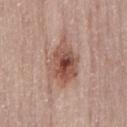workup = no biopsy performed (imaged during a skin exam) | patient = female, approximately 50 years of age | imaging modality = ~15 mm tile from a whole-body skin photo | body site = the lower back | size = about 6 mm | tile lighting = white-light.